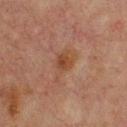Part of a total-body skin-imaging series; this lesion was reviewed on a skin check and was not flagged for biopsy.
A male patient aged around 65.
This image is a 15 mm lesion crop taken from a total-body photograph.
The lesion is on the back.
Imaged with cross-polarized lighting.